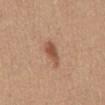Impression:
The lesion was tiled from a total-body skin photograph and was not biopsied.
Context:
Approximately 3.5 mm at its widest. The lesion-visualizer software estimated a footprint of about 4.5 mm², an outline eccentricity of about 0.85 (0 = round, 1 = elongated), and two-axis asymmetry of about 0.3. The analysis additionally found a normalized border contrast of about 8. And it measured a border-irregularity index near 3/10, internal color variation of about 2.5 on a 0–10 scale, and peripheral color asymmetry of about 1. The analysis additionally found a nevus-likeness score of about 85/100 and lesion-presence confidence of about 100/100. Located on the front of the torso. A female subject, about 55 years old. This is a white-light tile. A 15 mm crop from a total-body photograph taken for skin-cancer surveillance.